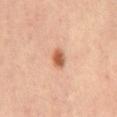The patient is a male aged 58–62. Cropped from a whole-body photographic skin survey; the tile spans about 15 mm. Approximately 2.5 mm at its widest. Located on the mid back. The tile uses cross-polarized illumination.